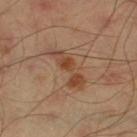patient:
  sex: male
  age_approx: 45
lesion_size:
  long_diameter_mm_approx: 6.0
image:
  source: total-body photography crop
  field_of_view_mm: 15
lighting: cross-polarized
automated_metrics:
  cielab_L: 44
  cielab_a: 21
  cielab_b: 31
  vs_skin_darker_L: 9.0
site: left thigh